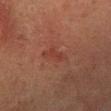The lesion was photographed on a routine skin check and not biopsied; there is no pathology result.
The lesion is located on the left lower leg.
A roughly 15 mm field-of-view crop from a total-body skin photograph.
A female patient, aged around 50.